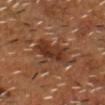Part of a total-body skin-imaging series; this lesion was reviewed on a skin check and was not flagged for biopsy. The recorded lesion diameter is about 5 mm. The lesion-visualizer software estimated a footprint of about 13 mm² and two-axis asymmetry of about 0.25. It also reported a color-variation rating of about 4.5/10 and peripheral color asymmetry of about 1.5. The analysis additionally found a nevus-likeness score of about 75/100. The tile uses cross-polarized illumination. Cropped from a whole-body photographic skin survey; the tile spans about 15 mm. A male subject, aged approximately 55. From the head or neck.